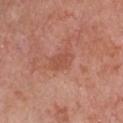Impression: This lesion was catalogued during total-body skin photography and was not selected for biopsy.